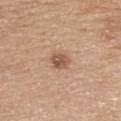Clinical impression:
Captured during whole-body skin photography for melanoma surveillance; the lesion was not biopsied.
Image and clinical context:
On the upper back. A 15 mm close-up extracted from a 3D total-body photography capture. This is a white-light tile. The patient is a female about 65 years old. Longest diameter approximately 2.5 mm.